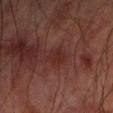Captured during whole-body skin photography for melanoma surveillance; the lesion was not biopsied. Imaged with cross-polarized lighting. The recorded lesion diameter is about 3 mm. A male subject roughly 65 years of age. A roughly 15 mm field-of-view crop from a total-body skin photograph. The lesion is located on the arm.